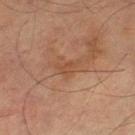follow-up = catalogued during a skin exam; not biopsied
location = the left thigh
lesion diameter = ≈3 mm
imaging modality = 15 mm crop, total-body photography
subject = male, aged 73 to 77
lighting = cross-polarized illumination